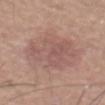The lesion was photographed on a routine skin check and not biopsied; there is no pathology result. The total-body-photography lesion software estimated a mean CIELAB color near L≈56 a*≈19 b*≈23, roughly 7 lightness units darker than nearby skin, and a normalized lesion–skin contrast near 5.5. It also reported a border-irregularity rating of about 3/10, a color-variation rating of about 3.5/10, and peripheral color asymmetry of about 1. Imaged with white-light lighting. Longest diameter approximately 9 mm. A roughly 15 mm field-of-view crop from a total-body skin photograph. The lesion is located on the front of the torso. A male subject, aged approximately 65.